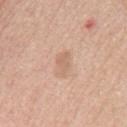Recorded during total-body skin imaging; not selected for excision or biopsy. From the mid back. Captured under white-light illumination. A female subject, aged 63–67. Automated image analysis of the tile measured an average lesion color of about L≈64 a*≈19 b*≈31 (CIELAB). And it measured a border-irregularity rating of about 2.5/10, internal color variation of about 1 on a 0–10 scale, and radial color variation of about 0.5. It also reported an automated nevus-likeness rating near 0 out of 100 and a lesion-detection confidence of about 100/100. About 3.5 mm across. A close-up tile cropped from a whole-body skin photograph, about 15 mm across.